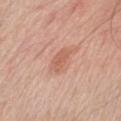Findings:
- follow-up — imaged on a skin check; not biopsied
- diameter — about 3 mm
- body site — the chest
- acquisition — total-body-photography crop, ~15 mm field of view
- illumination — white-light
- TBP lesion metrics — an area of roughly 4.5 mm² and two-axis asymmetry of about 0.25; a border-irregularity index near 2.5/10, a color-variation rating of about 2/10, and radial color variation of about 0.5; a lesion-detection confidence of about 100/100
- patient — male, aged 63 to 67